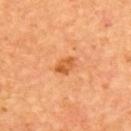Clinical impression:
No biopsy was performed on this lesion — it was imaged during a full skin examination and was not determined to be concerning.
Image and clinical context:
Captured under cross-polarized illumination. The lesion's longest dimension is about 2.5 mm. A 15 mm close-up tile from a total-body photography series done for melanoma screening. Located on the back. A male subject, about 65 years old.